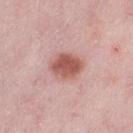| field | value |
|---|---|
| workup | no biopsy performed (imaged during a skin exam) |
| imaging modality | ~15 mm crop, total-body skin-cancer survey |
| patient | female, aged approximately 45 |
| anatomic site | the left thigh |